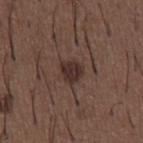Q: Was a biopsy performed?
A: imaged on a skin check; not biopsied